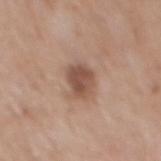| key | value |
|---|---|
| biopsy status | total-body-photography surveillance lesion; no biopsy |
| size | about 3.5 mm |
| image | total-body-photography crop, ~15 mm field of view |
| subject | male, roughly 60 years of age |
| location | the back |
| lighting | white-light |